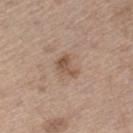<record>
  <biopsy_status>not biopsied; imaged during a skin examination</biopsy_status>
  <patient>
    <sex>male</sex>
    <age_approx>70</age_approx>
  </patient>
  <site>left thigh</site>
  <lesion_size>
    <long_diameter_mm_approx>3.0</long_diameter_mm_approx>
  </lesion_size>
  <image>
    <source>total-body photography crop</source>
    <field_of_view_mm>15</field_of_view_mm>
  </image>
</record>